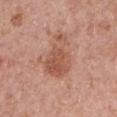Assessment:
Imaged during a routine full-body skin examination; the lesion was not biopsied and no histopathology is available.
Context:
The tile uses white-light illumination. Longest diameter approximately 6 mm. A roughly 15 mm field-of-view crop from a total-body skin photograph. The lesion is located on the right forearm. A female patient in their 40s.